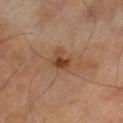Part of a total-body skin-imaging series; this lesion was reviewed on a skin check and was not flagged for biopsy.
Cropped from a whole-body photographic skin survey; the tile spans about 15 mm.
The lesion is located on the left thigh.
A male patient, approximately 70 years of age.